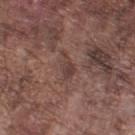biopsy_status: not biopsied; imaged during a skin examination
lesion_size:
  long_diameter_mm_approx: 3.5
image:
  source: total-body photography crop
  field_of_view_mm: 15
site: leg
lighting: white-light
patient:
  sex: male
  age_approx: 75